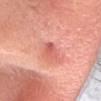  biopsy_status: not biopsied; imaged during a skin examination
  image:
    source: total-body photography crop
    field_of_view_mm: 15
  lesion_size:
    long_diameter_mm_approx: 3.0
  lighting: white-light
  patient:
    sex: female
    age_approx: 30
  site: head or neck